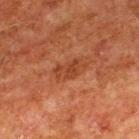Findings:
• biopsy status — imaged on a skin check; not biopsied
• patient — male, aged approximately 80
• site — the upper back
• imaging modality — 15 mm crop, total-body photography
• lighting — cross-polarized illumination
• diameter — ~3.5 mm (longest diameter)